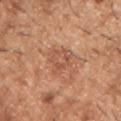workup = imaged on a skin check; not biopsied | subject = male, roughly 40 years of age | acquisition = ~15 mm crop, total-body skin-cancer survey | location = the chest.